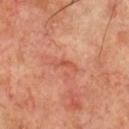  biopsy_status: not biopsied; imaged during a skin examination
  patient:
    sex: male
    age_approx: 70
  lighting: cross-polarized
  image:
    source: total-body photography crop
    field_of_view_mm: 15
  site: chest
  lesion_size:
    long_diameter_mm_approx: 3.0